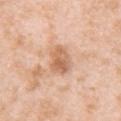biopsy status = imaged on a skin check; not biopsied
imaging modality = 15 mm crop, total-body photography
site = the arm
patient = female, aged around 40